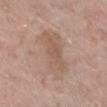Q: Was a biopsy performed?
A: imaged on a skin check; not biopsied
Q: What kind of image is this?
A: ~15 mm crop, total-body skin-cancer survey
Q: Lesion location?
A: the right lower leg
Q: What are the patient's age and sex?
A: female, in their mid- to late 50s
Q: What did automated image analysis measure?
A: an area of roughly 19 mm², a shape eccentricity near 0.85, and a symmetry-axis asymmetry near 0.3; a border-irregularity rating of about 3.5/10, internal color variation of about 3 on a 0–10 scale, and peripheral color asymmetry of about 1
Q: How large is the lesion?
A: ~7 mm (longest diameter)